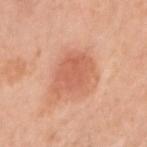Recorded during total-body skin imaging; not selected for excision or biopsy.
Measured at roughly 5 mm in maximum diameter.
The subject is a female aged 48 to 52.
Cropped from a total-body skin-imaging series; the visible field is about 15 mm.
On the right upper arm.